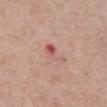| field | value |
|---|---|
| biopsy status | imaged on a skin check; not biopsied |
| illumination | white-light |
| patient | male, aged 73–77 |
| imaging modality | ~15 mm tile from a whole-body skin photo |
| anatomic site | the mid back |